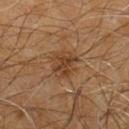No biopsy was performed on this lesion — it was imaged during a full skin examination and was not determined to be concerning. Captured under cross-polarized illumination. The lesion is on the chest. The patient is a male aged around 60. Cropped from a whole-body photographic skin survey; the tile spans about 15 mm. Longest diameter approximately 3.5 mm.